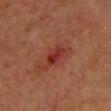biopsy status = no biopsy performed (imaged during a skin exam); lesion diameter = about 3.5 mm; imaging modality = ~15 mm crop, total-body skin-cancer survey; TBP lesion metrics = a lesion color around L≈29 a*≈28 b*≈25 in CIELAB; body site = the front of the torso; tile lighting = cross-polarized; subject = female, aged 53–57.